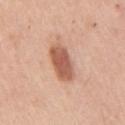Q: Was a biopsy performed?
A: total-body-photography surveillance lesion; no biopsy
Q: What are the patient's age and sex?
A: male, aged approximately 50
Q: What lighting was used for the tile?
A: white-light illumination
Q: Lesion location?
A: the right upper arm
Q: How was this image acquired?
A: total-body-photography crop, ~15 mm field of view
Q: Automated lesion metrics?
A: two-axis asymmetry of about 0.15; a lesion color around L≈58 a*≈24 b*≈31 in CIELAB, about 14 CIELAB-L* units darker than the surrounding skin, and a normalized lesion–skin contrast near 9; a border-irregularity rating of about 2/10 and a peripheral color-asymmetry measure near 1